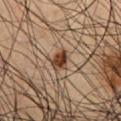Q: Was a biopsy performed?
A: catalogued during a skin exam; not biopsied
Q: What are the patient's age and sex?
A: male, about 50 years old
Q: Illumination type?
A: cross-polarized illumination
Q: Where on the body is the lesion?
A: the chest
Q: What is the imaging modality?
A: ~15 mm crop, total-body skin-cancer survey
Q: What did automated image analysis measure?
A: a mean CIELAB color near L≈38 a*≈19 b*≈28, roughly 16 lightness units darker than nearby skin, and a normalized border contrast of about 12.5; a border-irregularity rating of about 2.5/10 and a within-lesion color-variation index near 4.5/10
Q: How large is the lesion?
A: ≈2.5 mm